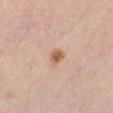Q: Is there a histopathology result?
A: no biopsy performed (imaged during a skin exam)
Q: What lighting was used for the tile?
A: white-light illumination
Q: Lesion location?
A: the front of the torso
Q: Patient demographics?
A: female, roughly 50 years of age
Q: What is the lesion's diameter?
A: ~2.5 mm (longest diameter)
Q: What is the imaging modality?
A: total-body-photography crop, ~15 mm field of view